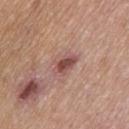Case summary:
– automated metrics — a classifier nevus-likeness of about 60/100 and lesion-presence confidence of about 100/100
– size — ~4 mm (longest diameter)
– location — the left thigh
– subject — female, approximately 60 years of age
– image source — total-body-photography crop, ~15 mm field of view
– tile lighting — white-light